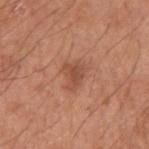| key | value |
|---|---|
| notes | total-body-photography surveillance lesion; no biopsy |
| image source | total-body-photography crop, ~15 mm field of view |
| automated metrics | an area of roughly 4.5 mm², an eccentricity of roughly 0.7, and a shape-asymmetry score of about 0.4 (0 = symmetric); a lesion color around L≈49 a*≈25 b*≈31 in CIELAB, a lesion–skin lightness drop of about 9, and a normalized border contrast of about 6.5; a border-irregularity index near 3.5/10, a color-variation rating of about 3/10, and a peripheral color-asymmetry measure near 1; a nevus-likeness score of about 55/100 and lesion-presence confidence of about 100/100 |
| illumination | white-light |
| lesion diameter | ~3 mm (longest diameter) |
| patient | male, about 65 years old |
| site | the right upper arm |